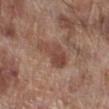Recorded during total-body skin imaging; not selected for excision or biopsy. A male subject about 70 years old. A 15 mm crop from a total-body photograph taken for skin-cancer surveillance. An algorithmic analysis of the crop reported a shape eccentricity near 0.9 and a shape-asymmetry score of about 0.4 (0 = symmetric). The analysis additionally found a border-irregularity index near 4.5/10, internal color variation of about 3.5 on a 0–10 scale, and peripheral color asymmetry of about 1. The lesion is located on the leg. Measured at roughly 5 mm in maximum diameter. This is a white-light tile.